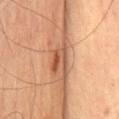Q: Where on the body is the lesion?
A: the chest
Q: How was the tile lit?
A: cross-polarized illumination
Q: How was this image acquired?
A: ~15 mm tile from a whole-body skin photo
Q: What is the lesion's diameter?
A: about 3.5 mm
Q: What are the patient's age and sex?
A: male, aged approximately 65
Q: Automated lesion metrics?
A: a symmetry-axis asymmetry near 0.4; a lesion–skin lightness drop of about 12 and a normalized border contrast of about 8.5; a border-irregularity index near 6.5/10, a color-variation rating of about 6/10, and peripheral color asymmetry of about 2; a nevus-likeness score of about 20/100 and a detector confidence of about 95 out of 100 that the crop contains a lesion